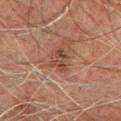Part of a total-body skin-imaging series; this lesion was reviewed on a skin check and was not flagged for biopsy.
The tile uses cross-polarized illumination.
The subject is a male approximately 75 years of age.
Longest diameter approximately 3 mm.
Automated image analysis of the tile measured a footprint of about 4 mm², an outline eccentricity of about 0.7 (0 = round, 1 = elongated), and two-axis asymmetry of about 0.45. And it measured an average lesion color of about L≈36 a*≈18 b*≈25 (CIELAB) and a normalized border contrast of about 7. And it measured border irregularity of about 5 on a 0–10 scale, a color-variation rating of about 2/10, and peripheral color asymmetry of about 0.
From the chest.
A 15 mm close-up extracted from a 3D total-body photography capture.